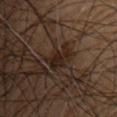biopsy status: no biopsy performed (imaged during a skin exam) | lesion diameter: ≈3.5 mm | acquisition: ~15 mm crop, total-body skin-cancer survey | anatomic site: the back | subject: male, in their 60s | tile lighting: cross-polarized.